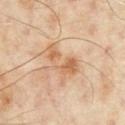Case summary:
* biopsy status: total-body-photography surveillance lesion; no biopsy
* image-analysis metrics: a lesion color around L≈61 a*≈19 b*≈35 in CIELAB and a normalized lesion–skin contrast near 6.5
* illumination: cross-polarized
* body site: the chest
* image source: ~15 mm crop, total-body skin-cancer survey
* patient: male, approximately 65 years of age